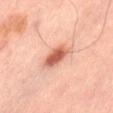notes=imaged on a skin check; not biopsied
subject=male, about 65 years old
imaging modality=total-body-photography crop, ~15 mm field of view
lesion diameter=~3.5 mm (longest diameter)
site=the left thigh
TBP lesion metrics=a shape eccentricity near 0.8; an average lesion color of about L≈59 a*≈28 b*≈32 (CIELAB), about 15 CIELAB-L* units darker than the surrounding skin, and a normalized border contrast of about 9.5; a nevus-likeness score of about 100/100
tile lighting=cross-polarized